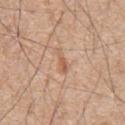workup: imaged on a skin check; not biopsied | imaging modality: 15 mm crop, total-body photography | lesion size: ≈3 mm | illumination: white-light illumination | TBP lesion metrics: a footprint of about 3 mm², an eccentricity of roughly 0.9, and a symmetry-axis asymmetry near 0.45; a lesion color around L≈60 a*≈20 b*≈33 in CIELAB; a classifier nevus-likeness of about 0/100 and a detector confidence of about 100 out of 100 that the crop contains a lesion | site: the abdomen | patient: male, aged 68–72.